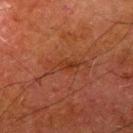biopsy status: total-body-photography surveillance lesion; no biopsy | location: the left upper arm | acquisition: ~15 mm tile from a whole-body skin photo | illumination: cross-polarized illumination | subject: male, roughly 80 years of age | lesion diameter: ~3.5 mm (longest diameter).